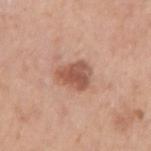Captured during whole-body skin photography for melanoma surveillance; the lesion was not biopsied. A male subject, aged 73–77. A lesion tile, about 15 mm wide, cut from a 3D total-body photograph. Approximately 4 mm at its widest. On the arm. The tile uses white-light illumination.